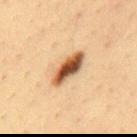Acquisition and patient details:
Captured under cross-polarized illumination. This image is a 15 mm lesion crop taken from a total-body photograph. An algorithmic analysis of the crop reported a footprint of about 8.5 mm², an eccentricity of roughly 0.9, and a symmetry-axis asymmetry near 0.15. It also reported a lesion color around L≈51 a*≈20 b*≈35 in CIELAB, a lesion–skin lightness drop of about 20, and a normalized border contrast of about 13. And it measured internal color variation of about 8.5 on a 0–10 scale and peripheral color asymmetry of about 2.5. Measured at roughly 5 mm in maximum diameter. A male subject aged around 35. The lesion is on the mid back.